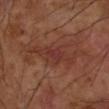Part of a total-body skin-imaging series; this lesion was reviewed on a skin check and was not flagged for biopsy. A male subject roughly 70 years of age. Captured under cross-polarized illumination. The lesion's longest dimension is about 8.5 mm. A region of skin cropped from a whole-body photographic capture, roughly 15 mm wide. Located on the left forearm.